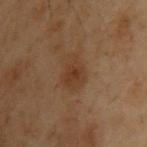The lesion was photographed on a routine skin check and not biopsied; there is no pathology result. The lesion is on the back. A male subject, aged around 55. A roughly 15 mm field-of-view crop from a total-body skin photograph. Approximately 4 mm at its widest. Imaged with cross-polarized lighting.